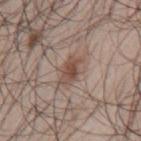| field | value |
|---|---|
| site | the mid back |
| illumination | white-light |
| automated metrics | a footprint of about 6.5 mm², an eccentricity of roughly 0.85, and a symmetry-axis asymmetry near 0.3; a color-variation rating of about 4/10 and a peripheral color-asymmetry measure near 1 |
| subject | male, aged 48 to 52 |
| size | about 4 mm |
| imaging modality | ~15 mm tile from a whole-body skin photo |